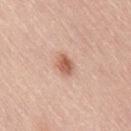Recorded during total-body skin imaging; not selected for excision or biopsy. Measured at roughly 2.5 mm in maximum diameter. A 15 mm close-up extracted from a 3D total-body photography capture. The patient is a female approximately 65 years of age. The lesion is on the right thigh.